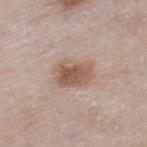Assessment:
This lesion was catalogued during total-body skin photography and was not selected for biopsy.
Acquisition and patient details:
Cropped from a total-body skin-imaging series; the visible field is about 15 mm. The patient is a male aged approximately 80. The lesion is located on the right lower leg. The lesion's longest dimension is about 4 mm. This is a white-light tile.